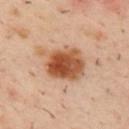Impression:
Imaged during a routine full-body skin examination; the lesion was not biopsied and no histopathology is available.
Clinical summary:
A region of skin cropped from a whole-body photographic capture, roughly 15 mm wide. The subject is a male aged around 40. The tile uses cross-polarized illumination. The lesion is on the upper back.